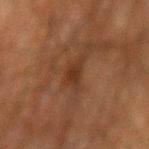Captured during whole-body skin photography for melanoma surveillance; the lesion was not biopsied.
Imaged with cross-polarized lighting.
A male patient aged 78 to 82.
A roughly 15 mm field-of-view crop from a total-body skin photograph.
On the mid back.
The recorded lesion diameter is about 3 mm.